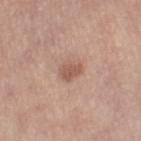Recorded during total-body skin imaging; not selected for excision or biopsy. From the right thigh. The lesion-visualizer software estimated a footprint of about 5 mm² and a shape eccentricity near 0.6. And it measured a lesion color around L≈56 a*≈20 b*≈28 in CIELAB, about 10 CIELAB-L* units darker than the surrounding skin, and a normalized border contrast of about 6.5. The analysis additionally found a border-irregularity index near 2/10, internal color variation of about 2 on a 0–10 scale, and a peripheral color-asymmetry measure near 0.5. Measured at roughly 3 mm in maximum diameter. Cropped from a total-body skin-imaging series; the visible field is about 15 mm. A female subject in their mid-60s.